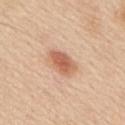Findings:
• notes — total-body-photography surveillance lesion; no biopsy
• image — ~15 mm crop, total-body skin-cancer survey
• patient — male, aged around 60
• site — the upper back